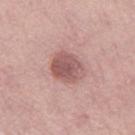Captured during whole-body skin photography for melanoma surveillance; the lesion was not biopsied. The lesion is located on the left thigh. Imaged with white-light lighting. The subject is a female aged 63–67. A 15 mm close-up tile from a total-body photography series done for melanoma screening.